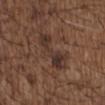Findings:
* biopsy status: total-body-photography surveillance lesion; no biopsy
* lighting: white-light
* image: ~15 mm tile from a whole-body skin photo
* subject: male, about 50 years old
* lesion size: about 5.5 mm
* anatomic site: the chest